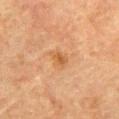Clinical impression:
The lesion was photographed on a routine skin check and not biopsied; there is no pathology result.
Acquisition and patient details:
Automated tile analysis of the lesion measured an average lesion color of about L≈46 a*≈19 b*≈34 (CIELAB), a lesion–skin lightness drop of about 6, and a normalized lesion–skin contrast near 6. A close-up tile cropped from a whole-body skin photograph, about 15 mm across. Located on the abdomen. A male subject roughly 80 years of age. The tile uses cross-polarized illumination. The lesion's longest dimension is about 2.5 mm.